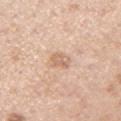Q: Was this lesion biopsied?
A: catalogued during a skin exam; not biopsied
Q: How large is the lesion?
A: ~2.5 mm (longest diameter)
Q: What kind of image is this?
A: ~15 mm crop, total-body skin-cancer survey
Q: How was the tile lit?
A: white-light
Q: Lesion location?
A: the right upper arm
Q: Patient demographics?
A: male, roughly 75 years of age